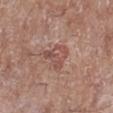The lesion was photographed on a routine skin check and not biopsied; there is no pathology result.
A close-up tile cropped from a whole-body skin photograph, about 15 mm across.
A female patient, in their mid-70s.
The lesion is located on the right lower leg.
Measured at roughly 3.5 mm in maximum diameter.